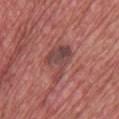subject = male, roughly 60 years of age; location = the upper back; lighting = white-light; lesion size = ~5 mm (longest diameter); acquisition = total-body-photography crop, ~15 mm field of view.